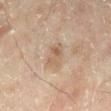No biopsy was performed on this lesion — it was imaged during a full skin examination and was not determined to be concerning. A close-up tile cropped from a whole-body skin photograph, about 15 mm across. The lesion is located on the left lower leg. Imaged with cross-polarized lighting. The patient is a male in their mid-40s. Approximately 3 mm at its widest.